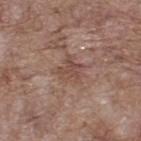notes — imaged on a skin check; not biopsied | imaging modality — total-body-photography crop, ~15 mm field of view | image-analysis metrics — a color-variation rating of about 3.5/10 and radial color variation of about 1.5; an automated nevus-likeness rating near 0 out of 100 and lesion-presence confidence of about 85/100 | size — about 3.5 mm | subject — male, aged 68 to 72 | site — the upper back.